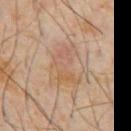No biopsy was performed on this lesion — it was imaged during a full skin examination and was not determined to be concerning. The lesion's longest dimension is about 5 mm. A male subject aged 58–62. A roughly 15 mm field-of-view crop from a total-body skin photograph. This is a cross-polarized tile. The lesion is on the abdomen.